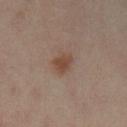Assessment:
Imaged during a routine full-body skin examination; the lesion was not biopsied and no histopathology is available.